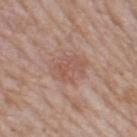Notes:
– size — ≈4.5 mm
– acquisition — total-body-photography crop, ~15 mm field of view
– illumination — white-light illumination
– location — the left thigh
– patient — female, aged around 60